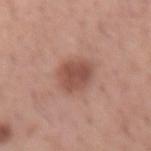biopsy status: catalogued during a skin exam; not biopsied | illumination: white-light | subject: male, aged approximately 40 | TBP lesion metrics: a footprint of about 9.5 mm², an eccentricity of roughly 0.6, and a shape-asymmetry score of about 0.15 (0 = symmetric); a border-irregularity index near 1.5/10, internal color variation of about 3 on a 0–10 scale, and a peripheral color-asymmetry measure near 1 | lesion size: ~4 mm (longest diameter) | acquisition: ~15 mm tile from a whole-body skin photo | body site: the right forearm.